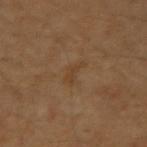Assessment: No biopsy was performed on this lesion — it was imaged during a full skin examination and was not determined to be concerning.